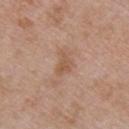Located on the chest. Imaged with white-light lighting. The total-body-photography lesion software estimated a lesion color around L≈55 a*≈19 b*≈31 in CIELAB, about 7 CIELAB-L* units darker than the surrounding skin, and a normalized border contrast of about 6. The analysis additionally found border irregularity of about 4 on a 0–10 scale, a within-lesion color-variation index near 2/10, and a peripheral color-asymmetry measure near 0.5. And it measured a nevus-likeness score of about 0/100. The subject is a male approximately 65 years of age. A roughly 15 mm field-of-view crop from a total-body skin photograph.